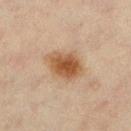Case summary:
- notes — no biopsy performed (imaged during a skin exam)
- tile lighting — cross-polarized
- subject — female, roughly 45 years of age
- imaging modality — ~15 mm crop, total-body skin-cancer survey
- anatomic site — the left lower leg